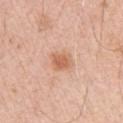The lesion is located on the left upper arm.
The lesion-visualizer software estimated a mean CIELAB color near L≈63 a*≈24 b*≈34, roughly 10 lightness units darker than nearby skin, and a normalized border contrast of about 7. And it measured a border-irregularity index near 2/10 and a within-lesion color-variation index near 2.5/10. It also reported a classifier nevus-likeness of about 75/100 and a lesion-detection confidence of about 100/100.
Imaged with white-light lighting.
The lesion's longest dimension is about 2.5 mm.
The subject is a male aged approximately 70.
A 15 mm crop from a total-body photograph taken for skin-cancer surveillance.